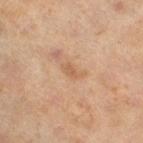Notes:
• body site: the right thigh
• patient: female, aged approximately 60
• acquisition: total-body-photography crop, ~15 mm field of view